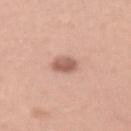biopsy status = no biopsy performed (imaged during a skin exam) | anatomic site = the left upper arm | image source = 15 mm crop, total-body photography | lesion diameter = ≈3 mm | automated lesion analysis = a color-variation rating of about 3.5/10 and peripheral color asymmetry of about 1; a classifier nevus-likeness of about 65/100 and a detector confidence of about 100 out of 100 that the crop contains a lesion | patient = female, aged approximately 45 | tile lighting = white-light illumination.